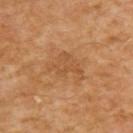No biopsy was performed on this lesion — it was imaged during a full skin examination and was not determined to be concerning. This is a cross-polarized tile. The total-body-photography lesion software estimated about 6 CIELAB-L* units darker than the surrounding skin and a normalized border contrast of about 5. A male subject, in their mid- to late 50s. Approximately 4.5 mm at its widest. A 15 mm close-up extracted from a 3D total-body photography capture. The lesion is on the upper back.